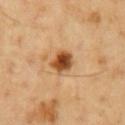Background:
Located on the chest. A close-up tile cropped from a whole-body skin photograph, about 15 mm across. Measured at roughly 3 mm in maximum diameter. The subject is a male aged 48 to 52.